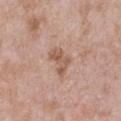Impression: Captured during whole-body skin photography for melanoma surveillance; the lesion was not biopsied. Context: The tile uses white-light illumination. About 3.5 mm across. From the front of the torso. A close-up tile cropped from a whole-body skin photograph, about 15 mm across. A male subject, aged around 50.